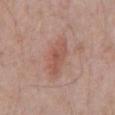Assessment:
The lesion was tiled from a total-body skin photograph and was not biopsied.
Image and clinical context:
The recorded lesion diameter is about 5 mm. Cropped from a whole-body photographic skin survey; the tile spans about 15 mm. A male patient, about 70 years old. The lesion is on the chest. This is a white-light tile.